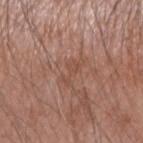biopsy status=total-body-photography surveillance lesion; no biopsy
lesion size=~3.5 mm (longest diameter)
acquisition=~15 mm crop, total-body skin-cancer survey
tile lighting=white-light illumination
patient=male, in their 60s
anatomic site=the right forearm